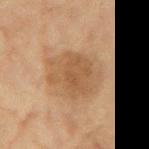patient: female, roughly 80 years of age
site: the left forearm
lighting: cross-polarized illumination
image source: ~15 mm tile from a whole-body skin photo